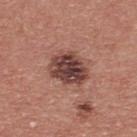Impression: No biopsy was performed on this lesion — it was imaged during a full skin examination and was not determined to be concerning. Clinical summary: The tile uses white-light illumination. Measured at roughly 4 mm in maximum diameter. The patient is a male aged 38–42. Located on the back. The total-body-photography lesion software estimated an area of roughly 12 mm², an eccentricity of roughly 0.6, and a symmetry-axis asymmetry near 0.15. The analysis additionally found an average lesion color of about L≈41 a*≈22 b*≈23 (CIELAB), about 16 CIELAB-L* units darker than the surrounding skin, and a normalized border contrast of about 12. And it measured border irregularity of about 1.5 on a 0–10 scale, a color-variation rating of about 6.5/10, and a peripheral color-asymmetry measure near 2.5. The software also gave an automated nevus-likeness rating near 20 out of 100 and a lesion-detection confidence of about 100/100. A close-up tile cropped from a whole-body skin photograph, about 15 mm across.